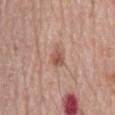Impression:
Captured during whole-body skin photography for melanoma surveillance; the lesion was not biopsied.
Context:
Automated tile analysis of the lesion measured an area of roughly 4.5 mm², an eccentricity of roughly 0.8, and two-axis asymmetry of about 0.25. It also reported a mean CIELAB color near L≈55 a*≈21 b*≈28. And it measured a border-irregularity rating of about 2.5/10, a color-variation rating of about 4.5/10, and a peripheral color-asymmetry measure near 1.5. Located on the back. The tile uses white-light illumination. A roughly 15 mm field-of-view crop from a total-body skin photograph. The lesion's longest dimension is about 3 mm. The subject is a male aged 78 to 82.